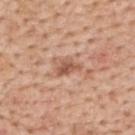Part of a total-body skin-imaging series; this lesion was reviewed on a skin check and was not flagged for biopsy.
From the back.
Measured at roughly 3 mm in maximum diameter.
A female subject roughly 50 years of age.
A 15 mm close-up tile from a total-body photography series done for melanoma screening.
An algorithmic analysis of the crop reported an area of roughly 4.5 mm², an eccentricity of roughly 0.8, and a shape-asymmetry score of about 0.35 (0 = symmetric). The analysis additionally found a lesion color around L≈56 a*≈22 b*≈31 in CIELAB, roughly 11 lightness units darker than nearby skin, and a normalized border contrast of about 7.5. The analysis additionally found a classifier nevus-likeness of about 0/100 and a detector confidence of about 100 out of 100 that the crop contains a lesion.
Imaged with white-light lighting.